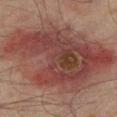Q: What kind of image is this?
A: total-body-photography crop, ~15 mm field of view
Q: What is the anatomic site?
A: the left lower leg
Q: What is the lesion's diameter?
A: ~13 mm (longest diameter)
Q: Automated lesion metrics?
A: a lesion color around L≈37 a*≈19 b*≈21 in CIELAB and about 10 CIELAB-L* units darker than the surrounding skin; a border-irregularity index near 3.5/10; a nevus-likeness score of about 0/100 and a lesion-detection confidence of about 100/100
Q: How was the tile lit?
A: cross-polarized illumination
Q: What are the patient's age and sex?
A: male, aged approximately 75